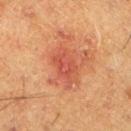This lesion was catalogued during total-body skin photography and was not selected for biopsy. The lesion is on the left lower leg. Captured under cross-polarized illumination. The recorded lesion diameter is about 4 mm. The subject is a male aged 58–62. Cropped from a total-body skin-imaging series; the visible field is about 15 mm.